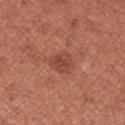This is a white-light tile. The lesion is on the right forearm. A roughly 15 mm field-of-view crop from a total-body skin photograph. A female patient, in their 50s. The recorded lesion diameter is about 3 mm. The lesion-visualizer software estimated a footprint of about 6 mm², a shape eccentricity near 0.5, and two-axis asymmetry of about 0.25. The software also gave a mean CIELAB color near L≈46 a*≈27 b*≈29, roughly 8 lightness units darker than nearby skin, and a lesion-to-skin contrast of about 6 (normalized; higher = more distinct).